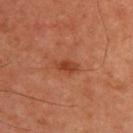No biopsy was performed on this lesion — it was imaged during a full skin examination and was not determined to be concerning. Longest diameter approximately 3 mm. The subject is a male aged approximately 50. The total-body-photography lesion software estimated an area of roughly 4 mm² and a symmetry-axis asymmetry near 0.3. And it measured a mean CIELAB color near L≈43 a*≈29 b*≈35. The software also gave border irregularity of about 2.5 on a 0–10 scale, internal color variation of about 2 on a 0–10 scale, and peripheral color asymmetry of about 0.5. The tile uses cross-polarized illumination. From the back. A lesion tile, about 15 mm wide, cut from a 3D total-body photograph.